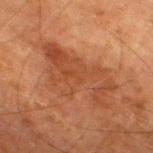Assessment:
This lesion was catalogued during total-body skin photography and was not selected for biopsy.
Background:
The tile uses cross-polarized illumination. The lesion is located on the leg. Longest diameter approximately 9 mm. Cropped from a whole-body photographic skin survey; the tile spans about 15 mm. The patient is a male about 80 years old.